The lesion is located on the front of the torso, the tile uses white-light illumination, The lesion-visualizer software estimated an average lesion color of about L≈45 a*≈21 b*≈24 (CIELAB) and roughly 11 lightness units darker than nearby skin. The analysis additionally found border irregularity of about 4.5 on a 0–10 scale and a color-variation rating of about 5.5/10, measured at roughly 4 mm in maximum diameter, a female patient in their mid-20s, this image is a 15 mm lesion crop taken from a total-body photograph:
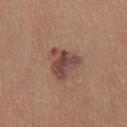Histopathologically confirmed as a nodular basal cell carcinoma.